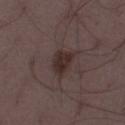biopsy_status: not biopsied; imaged during a skin examination
lighting: white-light
site: right thigh
image:
  source: total-body photography crop
  field_of_view_mm: 15
patient:
  sex: male
  age_approx: 50
lesion_size:
  long_diameter_mm_approx: 3.0
automated_metrics:
  border_irregularity_0_10: 2.0
  color_variation_0_10: 2.5
  peripheral_color_asymmetry: 1.0